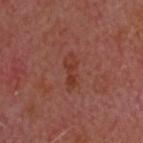The lesion was tiled from a total-body skin photograph and was not biopsied. A male subject roughly 65 years of age. Automated tile analysis of the lesion measured a shape eccentricity near 0.95. It also reported a mean CIELAB color near L≈37 a*≈26 b*≈29, roughly 6 lightness units darker than nearby skin, and a lesion-to-skin contrast of about 7 (normalized; higher = more distinct). And it measured a border-irregularity index near 5.5/10 and a peripheral color-asymmetry measure near 0.5. Longest diameter approximately 4 mm. Located on the head or neck. The tile uses cross-polarized illumination. A 15 mm close-up tile from a total-body photography series done for melanoma screening.